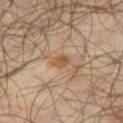notes=catalogued during a skin exam; not biopsied
imaging modality=15 mm crop, total-body photography
subject=male, roughly 65 years of age
body site=the right thigh